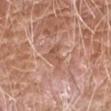location: the arm
patient: male, in their mid-70s
acquisition: 15 mm crop, total-body photography